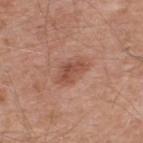{"biopsy_status": "not biopsied; imaged during a skin examination", "site": "upper back", "patient": {"sex": "male", "age_approx": 55}, "image": {"source": "total-body photography crop", "field_of_view_mm": 15}}